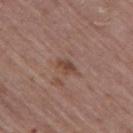Clinical summary:
From the left thigh. The tile uses white-light illumination. A close-up tile cropped from a whole-body skin photograph, about 15 mm across. An algorithmic analysis of the crop reported border irregularity of about 3 on a 0–10 scale and internal color variation of about 1.5 on a 0–10 scale. It also reported a nevus-likeness score of about 0/100. The recorded lesion diameter is about 3 mm. A male patient roughly 65 years of age.